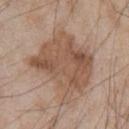biopsy status = no biopsy performed (imaged during a skin exam)
imaging modality = ~15 mm tile from a whole-body skin photo
location = the chest
patient = male, aged 63–67
size = ≈7.5 mm
lighting = white-light